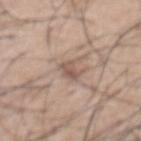Recorded during total-body skin imaging; not selected for excision or biopsy. A male subject roughly 55 years of age. Captured under white-light illumination. From the abdomen. About 3 mm across. A 15 mm close-up tile from a total-body photography series done for melanoma screening.